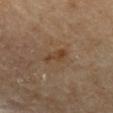follow-up=no biopsy performed (imaged during a skin exam)
lighting=cross-polarized
imaging modality=~15 mm crop, total-body skin-cancer survey
subject=male, aged approximately 85 or older
diameter=≈3 mm
anatomic site=the upper back